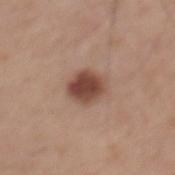follow-up: imaged on a skin check; not biopsied
illumination: white-light illumination
lesion diameter: about 3.5 mm
patient: male, about 65 years old
location: the mid back
image source: total-body-photography crop, ~15 mm field of view
automated lesion analysis: a footprint of about 9 mm² and two-axis asymmetry of about 0.15; an average lesion color of about L≈46 a*≈21 b*≈26 (CIELAB), roughly 14 lightness units darker than nearby skin, and a normalized lesion–skin contrast near 10.5; a border-irregularity rating of about 1.5/10 and a color-variation rating of about 5/10; a nevus-likeness score of about 95/100